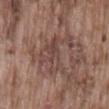workup=total-body-photography surveillance lesion; no biopsy.